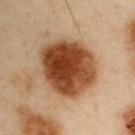{
  "biopsy_status": "not biopsied; imaged during a skin examination",
  "lesion_size": {
    "long_diameter_mm_approx": 7.0
  },
  "patient": {
    "sex": "male",
    "age_approx": 55
  },
  "site": "left upper arm",
  "image": {
    "source": "total-body photography crop",
    "field_of_view_mm": 15
  },
  "lighting": "cross-polarized"
}